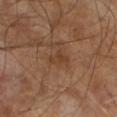{"biopsy_status": "not biopsied; imaged during a skin examination", "image": {"source": "total-body photography crop", "field_of_view_mm": 15}, "lighting": "cross-polarized", "patient": {"sex": "male", "age_approx": 65}}